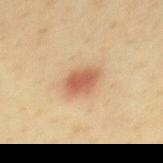Recorded during total-body skin imaging; not selected for excision or biopsy.